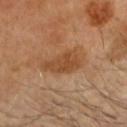patient: male, aged approximately 65
acquisition: ~15 mm tile from a whole-body skin photo
tile lighting: cross-polarized
location: the head or neck